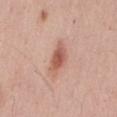Imaged during a routine full-body skin examination; the lesion was not biopsied and no histopathology is available. Longest diameter approximately 5 mm. A lesion tile, about 15 mm wide, cut from a 3D total-body photograph. The lesion is located on the mid back. A male subject aged 53 to 57.